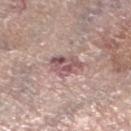biopsy status: imaged on a skin check; not biopsied | tile lighting: white-light | patient: female, aged 63 to 67 | lesion diameter: ~4 mm (longest diameter) | imaging modality: ~15 mm crop, total-body skin-cancer survey | anatomic site: the left lower leg.